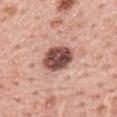biopsy status=catalogued during a skin exam; not biopsied
image source=15 mm crop, total-body photography
site=the back
TBP lesion metrics=border irregularity of about 1.5 on a 0–10 scale and peripheral color asymmetry of about 2; a lesion-detection confidence of about 100/100
subject=male, about 60 years old
lighting=white-light illumination
diameter=≈4 mm